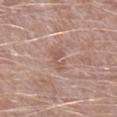A male patient in their 50s. Cropped from a total-body skin-imaging series; the visible field is about 15 mm. From the right lower leg. About 3 mm across. Captured under white-light illumination.